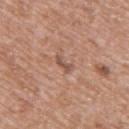site: the upper back; image: 15 mm crop, total-body photography; size: ~2.5 mm (longest diameter); tile lighting: white-light illumination; subject: female, aged 38 to 42.